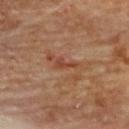| key | value |
|---|---|
| notes | total-body-photography surveillance lesion; no biopsy |
| size | about 2.5 mm |
| illumination | cross-polarized |
| location | the back |
| patient | male, in their mid-80s or older |
| imaging modality | ~15 mm tile from a whole-body skin photo |